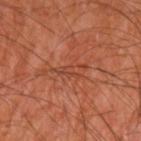Clinical impression: Captured during whole-body skin photography for melanoma surveillance; the lesion was not biopsied. Acquisition and patient details: This is a cross-polarized tile. The lesion-visualizer software estimated a lesion area of about 4.5 mm², an outline eccentricity of about 0.9 (0 = round, 1 = elongated), and a shape-asymmetry score of about 0.65 (0 = symmetric). The subject is a male aged 58–62. A 15 mm close-up extracted from a 3D total-body photography capture. The lesion's longest dimension is about 4 mm. Located on the left upper arm.